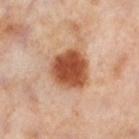A female patient, in their mid-50s. On the left lower leg. A close-up tile cropped from a whole-body skin photograph, about 15 mm across.